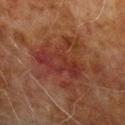lesion size: ~6 mm (longest diameter)
body site: the left upper arm
patient: male, aged 68 to 72
illumination: cross-polarized illumination
imaging modality: 15 mm crop, total-body photography
TBP lesion metrics: a footprint of about 22 mm², an outline eccentricity of about 0.25 (0 = round, 1 = elongated), and two-axis asymmetry of about 0.55; a lesion color around L≈29 a*≈23 b*≈24 in CIELAB, about 6 CIELAB-L* units darker than the surrounding skin, and a normalized border contrast of about 6.5; a border-irregularity index near 9/10, a within-lesion color-variation index near 6/10, and peripheral color asymmetry of about 2; a nevus-likeness score of about 0/100